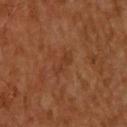Clinical impression:
The lesion was tiled from a total-body skin photograph and was not biopsied.
Clinical summary:
A male patient, aged 58–62. Captured under cross-polarized illumination. The lesion is located on the upper back. Automated image analysis of the tile measured a lesion area of about 4 mm². The software also gave an average lesion color of about L≈33 a*≈22 b*≈30 (CIELAB), about 5 CIELAB-L* units darker than the surrounding skin, and a lesion-to-skin contrast of about 4.5 (normalized; higher = more distinct). And it measured a border-irregularity rating of about 4/10, a within-lesion color-variation index near 0.5/10, and a peripheral color-asymmetry measure near 0. It also reported a nevus-likeness score of about 0/100 and a detector confidence of about 100 out of 100 that the crop contains a lesion. Cropped from a total-body skin-imaging series; the visible field is about 15 mm.